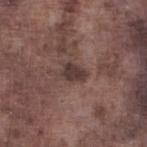Q: Was a biopsy performed?
A: no biopsy performed (imaged during a skin exam)
Q: What is the anatomic site?
A: the left lower leg
Q: How large is the lesion?
A: ~2.5 mm (longest diameter)
Q: Illumination type?
A: white-light illumination
Q: How was this image acquired?
A: 15 mm crop, total-body photography
Q: What are the patient's age and sex?
A: male, aged around 75